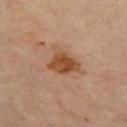The lesion was tiled from a total-body skin photograph and was not biopsied. The patient is a female aged 68–72. The lesion is on the left thigh. A close-up tile cropped from a whole-body skin photograph, about 15 mm across.